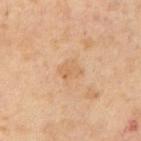Q: Was this lesion biopsied?
A: imaged on a skin check; not biopsied
Q: What kind of image is this?
A: ~15 mm tile from a whole-body skin photo
Q: How large is the lesion?
A: about 2.5 mm
Q: What are the patient's age and sex?
A: male, aged 63–67
Q: What lighting was used for the tile?
A: cross-polarized illumination
Q: What did automated image analysis measure?
A: an outline eccentricity of about 0.55 (0 = round, 1 = elongated) and a shape-asymmetry score of about 0.2 (0 = symmetric); an average lesion color of about L≈63 a*≈19 b*≈37 (CIELAB), roughly 6 lightness units darker than nearby skin, and a normalized border contrast of about 4.5